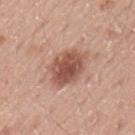The lesion was tiled from a total-body skin photograph and was not biopsied.
Measured at roughly 5 mm in maximum diameter.
The lesion is located on the left upper arm.
This is a white-light tile.
Automated tile analysis of the lesion measured a lesion color around L≈53 a*≈22 b*≈28 in CIELAB, a lesion–skin lightness drop of about 13, and a normalized border contrast of about 9. The analysis additionally found a border-irregularity rating of about 2/10, internal color variation of about 4.5 on a 0–10 scale, and a peripheral color-asymmetry measure near 1.5. The analysis additionally found a nevus-likeness score of about 85/100 and a detector confidence of about 100 out of 100 that the crop contains a lesion.
A region of skin cropped from a whole-body photographic capture, roughly 15 mm wide.
The subject is a male roughly 20 years of age.